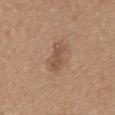The lesion was tiled from a total-body skin photograph and was not biopsied.